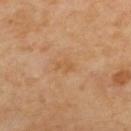follow-up = imaged on a skin check; not biopsied | lighting = cross-polarized illumination | acquisition = ~15 mm tile from a whole-body skin photo | diameter = about 3 mm | body site = the back.